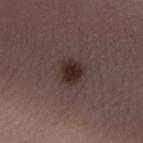Part of a total-body skin-imaging series; this lesion was reviewed on a skin check and was not flagged for biopsy. A female subject approximately 30 years of age. Imaged with white-light lighting. Approximately 3 mm at its widest. The total-body-photography lesion software estimated an eccentricity of roughly 0.4 and a symmetry-axis asymmetry near 0.2. It also reported a lesion–skin lightness drop of about 10 and a normalized border contrast of about 10.5. The lesion is on the arm. A 15 mm crop from a total-body photograph taken for skin-cancer surveillance.